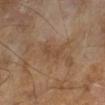Findings:
* biopsy status: total-body-photography surveillance lesion; no biopsy
* subject: male, aged approximately 65
* image: ~15 mm crop, total-body skin-cancer survey
* tile lighting: cross-polarized illumination
* diameter: ~3.5 mm (longest diameter)